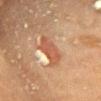follow-up — no biopsy performed (imaged during a skin exam) | patient — female, about 60 years old | anatomic site — the chest | image — ~15 mm crop, total-body skin-cancer survey | illumination — cross-polarized.